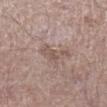workup — imaged on a skin check; not biopsied
automated metrics — a classifier nevus-likeness of about 0/100 and lesion-presence confidence of about 100/100
anatomic site — the right lower leg
patient — male, approximately 55 years of age
acquisition — ~15 mm crop, total-body skin-cancer survey